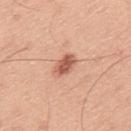Recorded during total-body skin imaging; not selected for excision or biopsy. Captured under white-light illumination. A male patient aged approximately 45. Cropped from a total-body skin-imaging series; the visible field is about 15 mm. Located on the upper back. About 3 mm across.